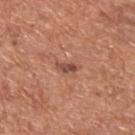Clinical impression: The lesion was photographed on a routine skin check and not biopsied; there is no pathology result. Background: Cropped from a total-body skin-imaging series; the visible field is about 15 mm. Located on the upper back. This is a white-light tile. A male subject aged around 65. The lesion-visualizer software estimated a nevus-likeness score of about 60/100. Measured at roughly 2.5 mm in maximum diameter.